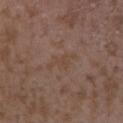biopsy status = no biopsy performed (imaged during a skin exam); body site = the left upper arm; acquisition = total-body-photography crop, ~15 mm field of view; size = about 4 mm; subject = female, aged around 35; illumination = white-light illumination.